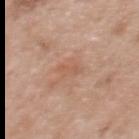Captured during whole-body skin photography for melanoma surveillance; the lesion was not biopsied. A female patient, aged approximately 50. Located on the upper back. Longest diameter approximately 3 mm. Cropped from a total-body skin-imaging series; the visible field is about 15 mm.